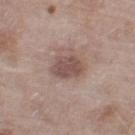<tbp_lesion>
<biopsy_status>not biopsied; imaged during a skin examination</biopsy_status>
<patient>
  <sex>female</sex>
  <age_approx>75</age_approx>
</patient>
<image>
  <source>total-body photography crop</source>
  <field_of_view_mm>15</field_of_view_mm>
</image>
<site>left thigh</site>
<automated_metrics>
  <color_variation_0_10>2.0</color_variation_0_10>
</automated_metrics>
</tbp_lesion>